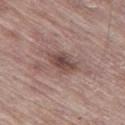{
  "biopsy_status": "not biopsied; imaged during a skin examination",
  "lesion_size": {
    "long_diameter_mm_approx": 3.5
  },
  "site": "left thigh",
  "patient": {
    "sex": "male",
    "age_approx": 65
  },
  "image": {
    "source": "total-body photography crop",
    "field_of_view_mm": 15
  },
  "lighting": "white-light"
}